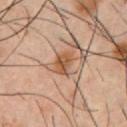This lesion was catalogued during total-body skin photography and was not selected for biopsy.
The subject is a male aged 38 to 42.
The lesion's longest dimension is about 3 mm.
This is a cross-polarized tile.
On the front of the torso.
A lesion tile, about 15 mm wide, cut from a 3D total-body photograph.